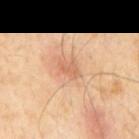| field | value |
|---|---|
| biopsy status | catalogued during a skin exam; not biopsied |
| patient | male, aged approximately 55 |
| body site | the back |
| image | total-body-photography crop, ~15 mm field of view |